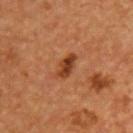No biopsy was performed on this lesion — it was imaged during a full skin examination and was not determined to be concerning. The lesion is on the chest. A 15 mm crop from a total-body photograph taken for skin-cancer surveillance. Imaged with cross-polarized lighting. A male subject aged approximately 55.